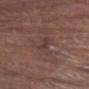{
  "site": "head or neck",
  "patient": {
    "sex": "male",
    "age_approx": 80
  },
  "image": {
    "source": "total-body photography crop",
    "field_of_view_mm": 15
  }
}